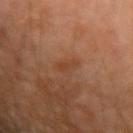Part of a total-body skin-imaging series; this lesion was reviewed on a skin check and was not flagged for biopsy.
Located on the left upper arm.
The patient is a male aged around 60.
A close-up tile cropped from a whole-body skin photograph, about 15 mm across.